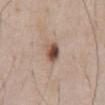Part of a total-body skin-imaging series; this lesion was reviewed on a skin check and was not flagged for biopsy. A male patient aged 58 to 62. Located on the front of the torso. The lesion-visualizer software estimated an area of roughly 4.5 mm², a shape eccentricity near 0.6, and a shape-asymmetry score of about 0.15 (0 = symmetric). And it measured an average lesion color of about L≈49 a*≈18 b*≈26 (CIELAB) and about 16 CIELAB-L* units darker than the surrounding skin. The analysis additionally found a classifier nevus-likeness of about 95/100 and lesion-presence confidence of about 100/100. A 15 mm close-up extracted from a 3D total-body photography capture. Imaged with white-light lighting.